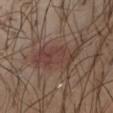- follow-up — imaged on a skin check; not biopsied
- anatomic site — the front of the torso
- tile lighting — white-light illumination
- size — ≈7 mm
- automated metrics — a footprint of about 14 mm², an outline eccentricity of about 0.9 (0 = round, 1 = elongated), and a symmetry-axis asymmetry near 0.35; a mean CIELAB color near L≈40 a*≈18 b*≈22 and a lesion–skin lightness drop of about 8; a border-irregularity index near 5.5/10, internal color variation of about 3.5 on a 0–10 scale, and a peripheral color-asymmetry measure near 1
- image source — ~15 mm crop, total-body skin-cancer survey
- patient — male, approximately 35 years of age